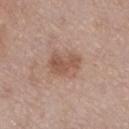Recorded during total-body skin imaging; not selected for excision or biopsy. A roughly 15 mm field-of-view crop from a total-body skin photograph. Located on the left lower leg. The total-body-photography lesion software estimated a classifier nevus-likeness of about 25/100 and lesion-presence confidence of about 100/100. A female subject in their mid-50s. This is a white-light tile.